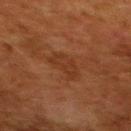Automated image analysis of the tile measured two-axis asymmetry of about 0.35. The analysis additionally found a lesion color around L≈29 a*≈20 b*≈28 in CIELAB and a lesion-to-skin contrast of about 5 (normalized; higher = more distinct). And it measured border irregularity of about 3.5 on a 0–10 scale and internal color variation of about 2 on a 0–10 scale. The software also gave an automated nevus-likeness rating near 0 out of 100 and a detector confidence of about 100 out of 100 that the crop contains a lesion.
From the upper back.
Longest diameter approximately 3.5 mm.
The tile uses cross-polarized illumination.
This image is a 15 mm lesion crop taken from a total-body photograph.
The subject is a female aged 48 to 52.